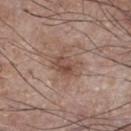The lesion was photographed on a routine skin check and not biopsied; there is no pathology result.